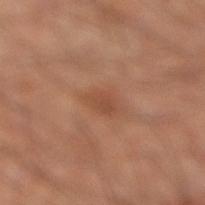<lesion>
  <lighting>cross-polarized</lighting>
  <site>right lower leg</site>
  <lesion_size>
    <long_diameter_mm_approx>2.5</long_diameter_mm_approx>
  </lesion_size>
  <image>
    <source>total-body photography crop</source>
    <field_of_view_mm>15</field_of_view_mm>
  </image>
  <patient>
    <sex>male</sex>
    <age_approx>60</age_approx>
  </patient>
</lesion>